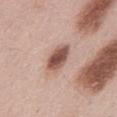Imaged during a routine full-body skin examination; the lesion was not biopsied and no histopathology is available. A male subject, aged approximately 55. The lesion is located on the mid back. A 15 mm crop from a total-body photograph taken for skin-cancer surveillance. Longest diameter approximately 3.5 mm. This is a white-light tile. Automated image analysis of the tile measured an average lesion color of about L≈52 a*≈21 b*≈25 (CIELAB), a lesion–skin lightness drop of about 17, and a lesion-to-skin contrast of about 11 (normalized; higher = more distinct). The software also gave a nevus-likeness score of about 95/100.